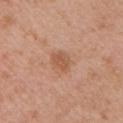Case summary:
• workup — total-body-photography surveillance lesion; no biopsy
• anatomic site — the chest
• subject — female, aged approximately 40
• imaging modality — total-body-photography crop, ~15 mm field of view
• tile lighting — white-light illumination
• TBP lesion metrics — a lesion color around L≈56 a*≈22 b*≈33 in CIELAB, roughly 8 lightness units darker than nearby skin, and a lesion-to-skin contrast of about 6 (normalized; higher = more distinct)
• lesion size — ~3 mm (longest diameter)